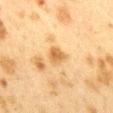<case>
<biopsy_status>not biopsied; imaged during a skin examination</biopsy_status>
<image>
  <source>total-body photography crop</source>
  <field_of_view_mm>15</field_of_view_mm>
</image>
<site>mid back</site>
<patient>
  <sex>female</sex>
  <age_approx>40</age_approx>
</patient>
</case>